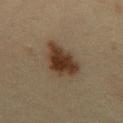Clinical impression:
The lesion was photographed on a routine skin check and not biopsied; there is no pathology result.
Clinical summary:
Automated image analysis of the tile measured a footprint of about 14 mm², an outline eccentricity of about 0.8 (0 = round, 1 = elongated), and two-axis asymmetry of about 0.3. The analysis additionally found a nevus-likeness score of about 100/100 and a detector confidence of about 100 out of 100 that the crop contains a lesion. About 6 mm across. A male patient, in their mid-30s. Imaged with cross-polarized lighting. The lesion is on the mid back. Cropped from a total-body skin-imaging series; the visible field is about 15 mm.